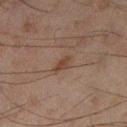Part of a total-body skin-imaging series; this lesion was reviewed on a skin check and was not flagged for biopsy.
On the left lower leg.
A region of skin cropped from a whole-body photographic capture, roughly 15 mm wide.
Automated image analysis of the tile measured a border-irregularity rating of about 4/10 and internal color variation of about 0.5 on a 0–10 scale. It also reported a detector confidence of about 100 out of 100 that the crop contains a lesion.
A male subject, approximately 45 years of age.
Approximately 2.5 mm at its widest.